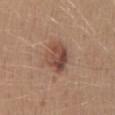Captured during whole-body skin photography for melanoma surveillance; the lesion was not biopsied. Measured at roughly 4 mm in maximum diameter. A female subject in their mid-40s. The total-body-photography lesion software estimated a border-irregularity index near 2/10 and peripheral color asymmetry of about 2.5. The lesion is on the lower back. Captured under white-light illumination. A 15 mm crop from a total-body photograph taken for skin-cancer surveillance.